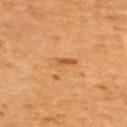{
  "biopsy_status": "not biopsied; imaged during a skin examination",
  "automated_metrics": {
    "area_mm2_approx": 4.5,
    "shape_asymmetry": 0.35,
    "border_irregularity_0_10": 4.0,
    "color_variation_0_10": 6.5,
    "peripheral_color_asymmetry": 2.5,
    "nevus_likeness_0_100": 85,
    "lesion_detection_confidence_0_100": 100
  },
  "site": "upper back",
  "lesion_size": {
    "long_diameter_mm_approx": 3.5
  },
  "image": {
    "source": "total-body photography crop",
    "field_of_view_mm": 15
  },
  "patient": {
    "sex": "male",
    "age_approx": 60
  }
}